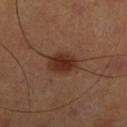biopsy status = imaged on a skin check; not biopsied
body site = the right lower leg
image-analysis metrics = a lesion–skin lightness drop of about 10
imaging modality = 15 mm crop, total-body photography
patient = male, aged 48 to 52
illumination = cross-polarized illumination
size = about 4 mm